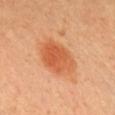Notes:
* biopsy status · no biopsy performed (imaged during a skin exam)
* image source · ~15 mm crop, total-body skin-cancer survey
* location · the head or neck
* automated lesion analysis · an average lesion color of about L≈59 a*≈31 b*≈42 (CIELAB) and a lesion–skin lightness drop of about 11
* patient · female, approximately 30 years of age